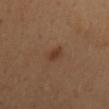The lesion was tiled from a total-body skin photograph and was not biopsied. Automated image analysis of the tile measured a lesion area of about 3 mm² and an outline eccentricity of about 0.75 (0 = round, 1 = elongated). The software also gave an average lesion color of about L≈36 a*≈19 b*≈29 (CIELAB), roughly 7 lightness units darker than nearby skin, and a normalized lesion–skin contrast near 7. And it measured border irregularity of about 2.5 on a 0–10 scale, internal color variation of about 1.5 on a 0–10 scale, and radial color variation of about 0.5. And it measured a classifier nevus-likeness of about 85/100 and a lesion-detection confidence of about 100/100. The recorded lesion diameter is about 2.5 mm. This is a cross-polarized tile. On the back. A male patient, in their mid- to late 60s. Cropped from a whole-body photographic skin survey; the tile spans about 15 mm.